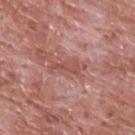Clinical impression: Imaged during a routine full-body skin examination; the lesion was not biopsied and no histopathology is available. Context: Measured at roughly 3.5 mm in maximum diameter. Automated tile analysis of the lesion measured an area of roughly 5 mm², an eccentricity of roughly 0.85, and a symmetry-axis asymmetry near 0.5. The analysis additionally found roughly 7 lightness units darker than nearby skin and a lesion-to-skin contrast of about 5.5 (normalized; higher = more distinct). It also reported a color-variation rating of about 1/10. A 15 mm close-up extracted from a 3D total-body photography capture. This is a white-light tile. Located on the upper back. A male patient aged 58 to 62.